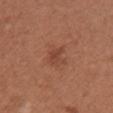Assessment:
The lesion was tiled from a total-body skin photograph and was not biopsied.
Clinical summary:
A lesion tile, about 15 mm wide, cut from a 3D total-body photograph. Automated tile analysis of the lesion measured a lesion area of about 4.5 mm², an outline eccentricity of about 0.5 (0 = round, 1 = elongated), and a symmetry-axis asymmetry near 0.4. The software also gave a lesion color around L≈44 a*≈24 b*≈31 in CIELAB, about 7 CIELAB-L* units darker than the surrounding skin, and a normalized lesion–skin contrast near 5.5. A female patient approximately 65 years of age. On the chest.